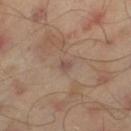biopsy status: total-body-photography surveillance lesion; no biopsy | diameter: about 1.5 mm | site: the left thigh | image: ~15 mm tile from a whole-body skin photo | patient: male, aged 43–47 | automated metrics: a lesion color around L≈47 a*≈17 b*≈23 in CIELAB, roughly 7 lightness units darker than nearby skin, and a normalized border contrast of about 6; a nevus-likeness score of about 0/100 and a detector confidence of about 100 out of 100 that the crop contains a lesion.